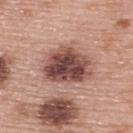Findings:
* follow-up · total-body-photography surveillance lesion; no biopsy
* imaging modality · ~15 mm crop, total-body skin-cancer survey
* illumination · white-light illumination
* lesion diameter · ≈6 mm
* anatomic site · the upper back
* patient · female, aged approximately 60
* automated metrics · an eccentricity of roughly 0.65 and a shape-asymmetry score of about 0.2 (0 = symmetric); a lesion color around L≈48 a*≈22 b*≈23 in CIELAB, roughly 17 lightness units darker than nearby skin, and a normalized border contrast of about 11.5; a nevus-likeness score of about 25/100